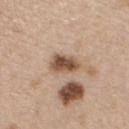  biopsy_status: not biopsied; imaged during a skin examination
  lesion_size:
    long_diameter_mm_approx: 3.5
  image:
    source: total-body photography crop
    field_of_view_mm: 15
  lighting: white-light
  patient:
    sex: female
    age_approx: 45
  automated_metrics:
    area_mm2_approx: 7.0
    eccentricity: 0.8
    shape_asymmetry: 0.15
    cielab_L: 52
    cielab_a: 18
    cielab_b: 31
    vs_skin_darker_L: 15.0
    vs_skin_contrast_norm: 10.5
  site: chest